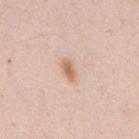Clinical impression:
The lesion was tiled from a total-body skin photograph and was not biopsied.
Context:
Automated tile analysis of the lesion measured an eccentricity of roughly 0.85 and a shape-asymmetry score of about 0.25 (0 = symmetric). The software also gave a lesion color around L≈65 a*≈20 b*≈31 in CIELAB, about 11 CIELAB-L* units darker than the surrounding skin, and a normalized lesion–skin contrast near 7.5. A male patient, aged around 35. On the mid back. The tile uses white-light illumination. Approximately 2.5 mm at its widest. A lesion tile, about 15 mm wide, cut from a 3D total-body photograph.